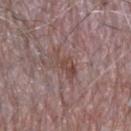Impression:
No biopsy was performed on this lesion — it was imaged during a full skin examination and was not determined to be concerning.
Clinical summary:
A lesion tile, about 15 mm wide, cut from a 3D total-body photograph. The tile uses white-light illumination. The lesion is located on the upper back. The lesion's longest dimension is about 4 mm. The patient is a male approximately 65 years of age.